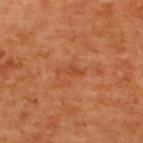{
  "biopsy_status": "not biopsied; imaged during a skin examination",
  "lesion_size": {
    "long_diameter_mm_approx": 3.0
  },
  "automated_metrics": {
    "shape_asymmetry": 0.5,
    "nevus_likeness_0_100": 0,
    "lesion_detection_confidence_0_100": 100
  },
  "patient": {
    "sex": "male",
    "age_approx": 65
  },
  "image": {
    "source": "total-body photography crop",
    "field_of_view_mm": 15
  },
  "site": "upper back"
}